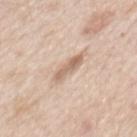Part of a total-body skin-imaging series; this lesion was reviewed on a skin check and was not flagged for biopsy. The lesion is on the mid back. A male patient, aged 58–62. Measured at roughly 4 mm in maximum diameter. Automated tile analysis of the lesion measured a lesion color around L≈65 a*≈16 b*≈29 in CIELAB, roughly 11 lightness units darker than nearby skin, and a normalized border contrast of about 7. It also reported a classifier nevus-likeness of about 10/100 and a lesion-detection confidence of about 95/100. Cropped from a total-body skin-imaging series; the visible field is about 15 mm. This is a white-light tile.